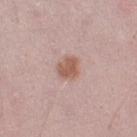notes = catalogued during a skin exam; not biopsied
image source = ~15 mm crop, total-body skin-cancer survey
TBP lesion metrics = a footprint of about 5.5 mm² and an eccentricity of roughly 0.6; a border-irregularity rating of about 2.5/10 and a within-lesion color-variation index near 2/10
subject = male, about 50 years old
body site = the right upper arm